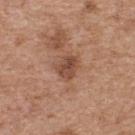<case>
<biopsy_status>not biopsied; imaged during a skin examination</biopsy_status>
<site>upper back</site>
<lesion_size>
  <long_diameter_mm_approx>3.0</long_diameter_mm_approx>
</lesion_size>
<automated_metrics>
  <cielab_L>47</cielab_L>
  <cielab_a>22</cielab_a>
  <cielab_b>29</cielab_b>
  <vs_skin_darker_L>10.0</vs_skin_darker_L>
  <vs_skin_contrast_norm>7.5</vs_skin_contrast_norm>
  <color_variation_0_10>3.5</color_variation_0_10>
  <peripheral_color_asymmetry>1.5</peripheral_color_asymmetry>
  <nevus_likeness_0_100>10</nevus_likeness_0_100>
  <lesion_detection_confidence_0_100>100</lesion_detection_confidence_0_100>
</automated_metrics>
<image>
  <source>total-body photography crop</source>
  <field_of_view_mm>15</field_of_view_mm>
</image>
<lighting>white-light</lighting>
<patient>
  <sex>female</sex>
  <age_approx>40</age_approx>
</patient>
</case>